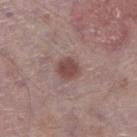  biopsy_status: not biopsied; imaged during a skin examination
  lighting: white-light
  image:
    source: total-body photography crop
    field_of_view_mm: 15
  automated_metrics:
    area_mm2_approx: 5.5
    shape_asymmetry: 0.15
    cielab_L: 46
    cielab_a: 20
    cielab_b: 22
    vs_skin_darker_L: 11.0
    vs_skin_contrast_norm: 8.5
    border_irregularity_0_10: 1.0
    color_variation_0_10: 1.5
    peripheral_color_asymmetry: 0.5
  lesion_size:
    long_diameter_mm_approx: 2.5
  site: leg
  patient:
    sex: male
    age_approx: 75